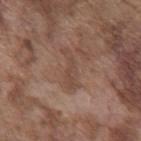Image and clinical context: The patient is a male roughly 75 years of age. A 15 mm close-up tile from a total-body photography series done for melanoma screening. Approximately 5 mm at its widest. Located on the chest. Automated tile analysis of the lesion measured a footprint of about 6 mm², an eccentricity of roughly 0.95, and a symmetry-axis asymmetry near 0.45. The software also gave a mean CIELAB color near L≈46 a*≈18 b*≈25, a lesion–skin lightness drop of about 6, and a normalized border contrast of about 5. The software also gave an automated nevus-likeness rating near 0 out of 100 and a lesion-detection confidence of about 90/100. Imaged with white-light lighting.